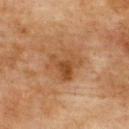The lesion was tiled from a total-body skin photograph and was not biopsied. Longest diameter approximately 5 mm. A male subject aged 73–77. A lesion tile, about 15 mm wide, cut from a 3D total-body photograph. Automated image analysis of the tile measured a mean CIELAB color near L≈40 a*≈19 b*≈32, about 8 CIELAB-L* units darker than the surrounding skin, and a normalized border contrast of about 7. The analysis additionally found a border-irregularity rating of about 4.5/10, a color-variation rating of about 5/10, and radial color variation of about 1.5. The software also gave a classifier nevus-likeness of about 20/100 and a lesion-detection confidence of about 100/100. The lesion is located on the upper back. This is a cross-polarized tile.